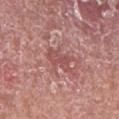Imaged during a routine full-body skin examination; the lesion was not biopsied and no histopathology is available. Imaged with white-light lighting. A male subject, aged approximately 80. A 15 mm crop from a total-body photograph taken for skin-cancer surveillance. The lesion is on the left lower leg.